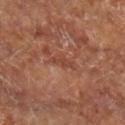Q: Is there a histopathology result?
A: no biopsy performed (imaged during a skin exam)
Q: Illumination type?
A: cross-polarized illumination
Q: Lesion location?
A: the right lower leg
Q: What did automated image analysis measure?
A: an area of roughly 2 mm², a shape eccentricity near 0.95, and a symmetry-axis asymmetry near 0.5; an average lesion color of about L≈42 a*≈24 b*≈29 (CIELAB), roughly 7 lightness units darker than nearby skin, and a normalized border contrast of about 6; a border-irregularity rating of about 5.5/10, a within-lesion color-variation index near 0/10, and a peripheral color-asymmetry measure near 0; a nevus-likeness score of about 0/100 and a detector confidence of about 70 out of 100 that the crop contains a lesion
Q: What is the lesion's diameter?
A: about 2.5 mm
Q: What are the patient's age and sex?
A: in their mid-60s
Q: How was this image acquired?
A: ~15 mm crop, total-body skin-cancer survey